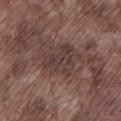Context: On the left lower leg. The lesion's longest dimension is about 4 mm. The lesion-visualizer software estimated an area of roughly 9.5 mm² and a shape eccentricity near 0.55. A roughly 15 mm field-of-view crop from a total-body skin photograph. The subject is a male about 75 years old.